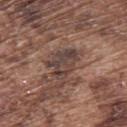<record>
  <biopsy_status>not biopsied; imaged during a skin examination</biopsy_status>
  <image>
    <source>total-body photography crop</source>
    <field_of_view_mm>15</field_of_view_mm>
  </image>
  <lighting>white-light</lighting>
  <site>back</site>
  <patient>
    <sex>male</sex>
    <age_approx>75</age_approx>
  </patient>
  <lesion_size>
    <long_diameter_mm_approx>4.0</long_diameter_mm_approx>
  </lesion_size>
</record>